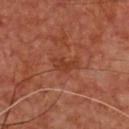follow-up: no biopsy performed (imaged during a skin exam)
tile lighting: cross-polarized illumination
lesion diameter: ≈3.5 mm
image source: ~15 mm crop, total-body skin-cancer survey
anatomic site: the chest
automated lesion analysis: an outline eccentricity of about 0.9 (0 = round, 1 = elongated) and a symmetry-axis asymmetry near 0.35; a lesion color around L≈37 a*≈26 b*≈32 in CIELAB, about 7 CIELAB-L* units darker than the surrounding skin, and a lesion-to-skin contrast of about 6 (normalized; higher = more distinct); a color-variation rating of about 1/10 and peripheral color asymmetry of about 0.5
patient: male, about 65 years old